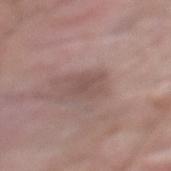The lesion was tiled from a total-body skin photograph and was not biopsied. From the mid back. A 15 mm close-up tile from a total-body photography series done for melanoma screening. The recorded lesion diameter is about 3 mm. Captured under white-light illumination. Automated tile analysis of the lesion measured border irregularity of about 2.5 on a 0–10 scale, internal color variation of about 1.5 on a 0–10 scale, and a peripheral color-asymmetry measure near 0.5. The software also gave a nevus-likeness score of about 0/100. A male patient roughly 60 years of age.